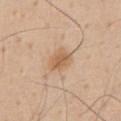{
  "biopsy_status": "not biopsied; imaged during a skin examination",
  "image": {
    "source": "total-body photography crop",
    "field_of_view_mm": 15
  },
  "lighting": "white-light",
  "automated_metrics": {
    "cielab_L": 61,
    "cielab_a": 18,
    "cielab_b": 35,
    "vs_skin_darker_L": 9.0,
    "vs_skin_contrast_norm": 7.0,
    "nevus_likeness_0_100": 65,
    "lesion_detection_confidence_0_100": 100
  },
  "lesion_size": {
    "long_diameter_mm_approx": 3.5
  },
  "site": "chest",
  "patient": {
    "sex": "male",
    "age_approx": 55
  }
}